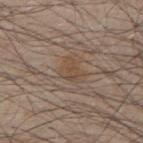size — ~3 mm (longest diameter)
image source — total-body-photography crop, ~15 mm field of view
illumination — white-light illumination
TBP lesion metrics — an area of roughly 5 mm² and a shape-asymmetry score of about 0.4 (0 = symmetric); an average lesion color of about L≈47 a*≈14 b*≈28 (CIELAB), about 5 CIELAB-L* units darker than the surrounding skin, and a normalized border contrast of about 5.5; border irregularity of about 4 on a 0–10 scale, a color-variation rating of about 2/10, and peripheral color asymmetry of about 1
subject — male, aged 43 to 47
body site — the upper back